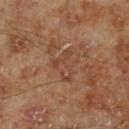The lesion was photographed on a routine skin check and not biopsied; there is no pathology result.
Automated tile analysis of the lesion measured an average lesion color of about L≈42 a*≈21 b*≈29 (CIELAB), roughly 6 lightness units darker than nearby skin, and a lesion-to-skin contrast of about 5 (normalized; higher = more distinct). The software also gave a nevus-likeness score of about 0/100 and lesion-presence confidence of about 80/100.
Located on the left lower leg.
The tile uses cross-polarized illumination.
A roughly 15 mm field-of-view crop from a total-body skin photograph.
The patient is a male aged 68 to 72.
Longest diameter approximately 4 mm.